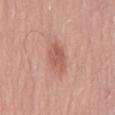No biopsy was performed on this lesion — it was imaged during a full skin examination and was not determined to be concerning. The lesion-visualizer software estimated an average lesion color of about L≈57 a*≈25 b*≈29 (CIELAB), a lesion–skin lightness drop of about 10, and a lesion-to-skin contrast of about 6.5 (normalized; higher = more distinct). It also reported a within-lesion color-variation index near 2.5/10 and radial color variation of about 1. Imaged with white-light lighting. The subject is a male in their mid-50s. A 15 mm close-up tile from a total-body photography series done for melanoma screening. The lesion is on the mid back.